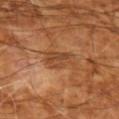notes=total-body-photography surveillance lesion; no biopsy
location=the left upper arm
image source=15 mm crop, total-body photography
patient=aged 63 to 67
image-analysis metrics=a footprint of about 8 mm² and a shape eccentricity near 0.7; a mean CIELAB color near L≈45 a*≈23 b*≈36, a lesion–skin lightness drop of about 8, and a normalized border contrast of about 6; a nevus-likeness score of about 10/100 and a lesion-detection confidence of about 100/100